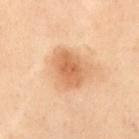Notes:
– workup — total-body-photography surveillance lesion; no biopsy
– imaging modality — total-body-photography crop, ~15 mm field of view
– subject — female, about 60 years old
– automated metrics — a footprint of about 11 mm² and an eccentricity of roughly 0.7; an average lesion color of about L≈56 a*≈21 b*≈35 (CIELAB) and roughly 10 lightness units darker than nearby skin; a nevus-likeness score of about 70/100 and a detector confidence of about 100 out of 100 that the crop contains a lesion
– size — about 4.5 mm
– lighting — cross-polarized
– location — the abdomen